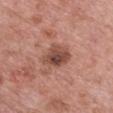This lesion was catalogued during total-body skin photography and was not selected for biopsy.
A female patient aged 58 to 62.
A region of skin cropped from a whole-body photographic capture, roughly 15 mm wide.
The lesion's longest dimension is about 3.5 mm.
From the chest.
Automated image analysis of the tile measured a peripheral color-asymmetry measure near 2.